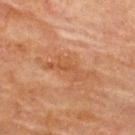<record>
<biopsy_status>not biopsied; imaged during a skin examination</biopsy_status>
<patient>
  <sex>female</sex>
  <age_approx>80</age_approx>
</patient>
<image>
  <source>total-body photography crop</source>
  <field_of_view_mm>15</field_of_view_mm>
</image>
<site>upper back</site>
</record>